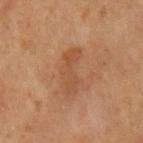follow-up: imaged on a skin check; not biopsied
body site: the back
image: 15 mm crop, total-body photography
illumination: cross-polarized illumination
image-analysis metrics: a footprint of about 8.5 mm², an outline eccentricity of about 0.95 (0 = round, 1 = elongated), and a symmetry-axis asymmetry near 0.45; a mean CIELAB color near L≈45 a*≈20 b*≈32, a lesion–skin lightness drop of about 6, and a normalized lesion–skin contrast near 5.5
subject: male, about 60 years old